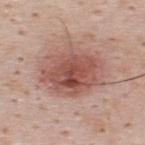Assessment: The lesion was tiled from a total-body skin photograph and was not biopsied. Clinical summary: On the back. This image is a 15 mm lesion crop taken from a total-body photograph. Captured under white-light illumination. About 6.5 mm across. A male subject aged 33–37. An algorithmic analysis of the crop reported a mean CIELAB color near L≈53 a*≈24 b*≈25 and a normalized lesion–skin contrast near 8.5. It also reported a classifier nevus-likeness of about 95/100 and a lesion-detection confidence of about 100/100.